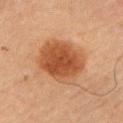Recorded during total-body skin imaging; not selected for excision or biopsy.
The subject is a male in their mid- to late 60s.
Automated image analysis of the tile measured a footprint of about 23 mm², an eccentricity of roughly 0.55, and a symmetry-axis asymmetry near 0.1. The software also gave a border-irregularity index near 1.5/10. It also reported a nevus-likeness score of about 100/100.
This image is a 15 mm lesion crop taken from a total-body photograph.
This is a cross-polarized tile.
Longest diameter approximately 5.5 mm.
Located on the right upper arm.